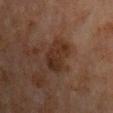{
  "biopsy_status": "not biopsied; imaged during a skin examination",
  "site": "upper back",
  "automated_metrics": {
    "eccentricity": 0.8,
    "shape_asymmetry": 0.25,
    "color_variation_0_10": 3.0,
    "peripheral_color_asymmetry": 1.0
  },
  "image": {
    "source": "total-body photography crop",
    "field_of_view_mm": 15
  },
  "lighting": "cross-polarized",
  "patient": {
    "sex": "male",
    "age_approx": 65
  }
}